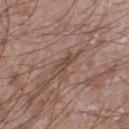Part of a total-body skin-imaging series; this lesion was reviewed on a skin check and was not flagged for biopsy. This is a white-light tile. Longest diameter approximately 4 mm. A roughly 15 mm field-of-view crop from a total-body skin photograph. The lesion is on the leg. The patient is a male aged approximately 60.